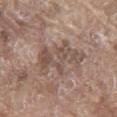{
  "automated_metrics": {
    "cielab_L": 50,
    "cielab_a": 15,
    "cielab_b": 23,
    "vs_skin_darker_L": 8.0
  },
  "lighting": "white-light",
  "patient": {
    "sex": "male",
    "age_approx": 80
  },
  "site": "chest",
  "image": {
    "source": "total-body photography crop",
    "field_of_view_mm": 15
  },
  "lesion_size": {
    "long_diameter_mm_approx": 6.0
  }
}